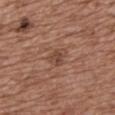Assessment: The lesion was tiled from a total-body skin photograph and was not biopsied. Background: The lesion is located on the upper back. A female subject roughly 75 years of age. Cropped from a total-body skin-imaging series; the visible field is about 15 mm.